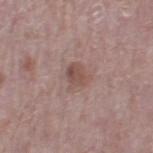Recorded during total-body skin imaging; not selected for excision or biopsy.
This is a white-light tile.
Cropped from a whole-body photographic skin survey; the tile spans about 15 mm.
Automated tile analysis of the lesion measured a symmetry-axis asymmetry near 0.3. It also reported a border-irregularity rating of about 3/10, internal color variation of about 3 on a 0–10 scale, and radial color variation of about 1. The analysis additionally found a classifier nevus-likeness of about 30/100 and a lesion-detection confidence of about 100/100.
The subject is a male about 70 years old.
Approximately 2.5 mm at its widest.
From the right thigh.